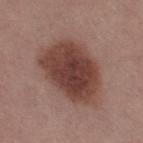Imaged during a routine full-body skin examination; the lesion was not biopsied and no histopathology is available. A lesion tile, about 15 mm wide, cut from a 3D total-body photograph. The lesion is on the right thigh. The recorded lesion diameter is about 7.5 mm. A female patient aged 48–52. Captured under white-light illumination.